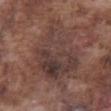Part of a total-body skin-imaging series; this lesion was reviewed on a skin check and was not flagged for biopsy.
This is a white-light tile.
Longest diameter approximately 8.5 mm.
The total-body-photography lesion software estimated a shape eccentricity near 0.7 and two-axis asymmetry of about 0.3. It also reported about 7 CIELAB-L* units darker than the surrounding skin and a normalized border contrast of about 7. The software also gave a nevus-likeness score of about 0/100 and a detector confidence of about 65 out of 100 that the crop contains a lesion.
From the abdomen.
The patient is a male aged 73–77.
This image is a 15 mm lesion crop taken from a total-body photograph.